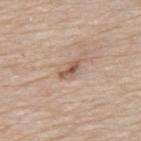biopsy_status: not biopsied; imaged during a skin examination
image:
  source: total-body photography crop
  field_of_view_mm: 15
patient:
  sex: male
  age_approx: 75
lighting: white-light
site: upper back